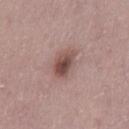Imaged during a routine full-body skin examination; the lesion was not biopsied and no histopathology is available. Located on the leg. A female subject aged 28–32. The lesion-visualizer software estimated a lesion–skin lightness drop of about 13 and a normalized border contrast of about 9.5. It also reported a border-irregularity index near 2/10 and internal color variation of about 5.5 on a 0–10 scale. About 3.5 mm across. This image is a 15 mm lesion crop taken from a total-body photograph.